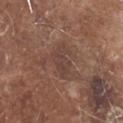The lesion is on the head or neck.
A 15 mm close-up tile from a total-body photography series done for melanoma screening.
A male patient aged around 80.